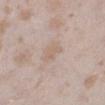<lesion>
  <biopsy_status>not biopsied; imaged during a skin examination</biopsy_status>
  <image>
    <source>total-body photography crop</source>
    <field_of_view_mm>15</field_of_view_mm>
  </image>
  <lesion_size>
    <long_diameter_mm_approx>3.0</long_diameter_mm_approx>
  </lesion_size>
  <patient>
    <sex>female</sex>
    <age_approx>25</age_approx>
  </patient>
  <site>left lower leg</site>
  <lighting>white-light</lighting>
  <automated_metrics>
    <cielab_L>63</cielab_L>
    <cielab_a>14</cielab_a>
    <cielab_b>27</cielab_b>
    <vs_skin_darker_L>6.0</vs_skin_darker_L>
    <vs_skin_contrast_norm>5.0</vs_skin_contrast_norm>
    <border_irregularity_0_10>3.5</border_irregularity_0_10>
    <color_variation_0_10>1.5</color_variation_0_10>
    <peripheral_color_asymmetry>0.5</peripheral_color_asymmetry>
    <nevus_likeness_0_100>0</nevus_likeness_0_100>
  </automated_metrics>
</lesion>